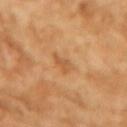Q: Was a biopsy performed?
A: imaged on a skin check; not biopsied
Q: What did automated image analysis measure?
A: a lesion area of about 3.5 mm², an outline eccentricity of about 0.75 (0 = round, 1 = elongated), and a shape-asymmetry score of about 0.4 (0 = symmetric); an automated nevus-likeness rating near 0 out of 100 and a lesion-detection confidence of about 100/100
Q: Who is the patient?
A: female, roughly 70 years of age
Q: What lighting was used for the tile?
A: cross-polarized
Q: Lesion size?
A: ≈2.5 mm
Q: What is the anatomic site?
A: the left upper arm
Q: How was this image acquired?
A: ~15 mm tile from a whole-body skin photo